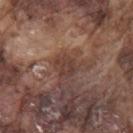notes: catalogued during a skin exam; not biopsied
imaging modality: total-body-photography crop, ~15 mm field of view
site: the left upper arm
diameter: about 2.5 mm
automated lesion analysis: border irregularity of about 2.5 on a 0–10 scale, a color-variation rating of about 2.5/10, and a peripheral color-asymmetry measure near 1
subject: male, in their mid-70s
lighting: white-light